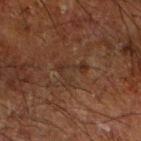workup=total-body-photography surveillance lesion; no biopsy
lighting=cross-polarized illumination
patient=male, aged 63 to 67
imaging modality=~15 mm crop, total-body skin-cancer survey
location=the right forearm